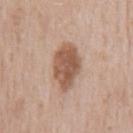Q: Was this lesion biopsied?
A: total-body-photography surveillance lesion; no biopsy
Q: What is the anatomic site?
A: the chest
Q: How was this image acquired?
A: ~15 mm tile from a whole-body skin photo
Q: What are the patient's age and sex?
A: male, about 65 years old